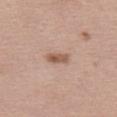Context:
Located on the left thigh. A 15 mm close-up tile from a total-body photography series done for melanoma screening. The patient is a female aged 38–42. The tile uses white-light illumination. The recorded lesion diameter is about 3 mm.